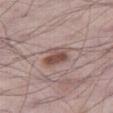  biopsy_status: not biopsied; imaged during a skin examination
  automated_metrics:
    cielab_L: 51
    cielab_a: 18
    cielab_b: 22
    vs_skin_darker_L: 11.0
    vs_skin_contrast_norm: 8.0
    border_irregularity_0_10: 2.5
    peripheral_color_asymmetry: 2.5
  site: leg
  lighting: white-light
  patient:
    sex: male
    age_approx: 70
  image:
    source: total-body photography crop
    field_of_view_mm: 15
  lesion_size:
    long_diameter_mm_approx: 3.5